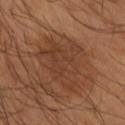The lesion was tiled from a total-body skin photograph and was not biopsied.
A male patient aged approximately 65.
The recorded lesion diameter is about 7 mm.
Captured under cross-polarized illumination.
On the right forearm.
This image is a 15 mm lesion crop taken from a total-body photograph.
The lesion-visualizer software estimated a lesion area of about 20 mm², an eccentricity of roughly 0.75, and two-axis asymmetry of about 0.4. And it measured a mean CIELAB color near L≈39 a*≈22 b*≈30, a lesion–skin lightness drop of about 6, and a normalized lesion–skin contrast near 5.5. It also reported a border-irregularity rating of about 6/10 and internal color variation of about 3.5 on a 0–10 scale.